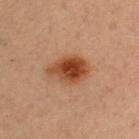Findings:
– workup · imaged on a skin check; not biopsied
– body site · the chest
– illumination · cross-polarized
– image · 15 mm crop, total-body photography
– patient · female, aged 38–42
– automated lesion analysis · a lesion area of about 11 mm² and a shape eccentricity near 0.75; a lesion color around L≈36 a*≈22 b*≈30 in CIELAB and about 12 CIELAB-L* units darker than the surrounding skin; a border-irregularity rating of about 2.5/10 and peripheral color asymmetry of about 1.5; a classifier nevus-likeness of about 100/100 and lesion-presence confidence of about 100/100
– diameter · ≈4.5 mm